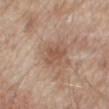Clinical impression: The lesion was photographed on a routine skin check and not biopsied; there is no pathology result. Image and clinical context: Cropped from a total-body skin-imaging series; the visible field is about 15 mm. A male patient, aged 78–82. The tile uses white-light illumination. The lesion is located on the mid back.